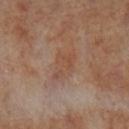The lesion was photographed on a routine skin check and not biopsied; there is no pathology result.
A female patient, roughly 70 years of age.
The lesion is located on the left lower leg.
A region of skin cropped from a whole-body photographic capture, roughly 15 mm wide.